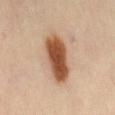Imaged during a routine full-body skin examination; the lesion was not biopsied and no histopathology is available. Cropped from a total-body skin-imaging series; the visible field is about 15 mm. From the lower back. The lesion's longest dimension is about 6 mm. A female subject, aged 38–42. Captured under cross-polarized illumination.